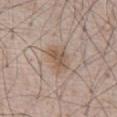Clinical impression: This lesion was catalogued during total-body skin photography and was not selected for biopsy. Clinical summary: This is a white-light tile. From the front of the torso. A male patient aged 63–67. A 15 mm crop from a total-body photograph taken for skin-cancer surveillance.